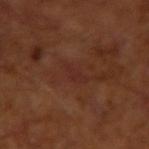No biopsy was performed on this lesion — it was imaged during a full skin examination and was not determined to be concerning. The total-body-photography lesion software estimated a lesion area of about 2.5 mm², an eccentricity of roughly 0.9, and a shape-asymmetry score of about 0.55 (0 = symmetric). And it measured border irregularity of about 6 on a 0–10 scale, a color-variation rating of about 0/10, and peripheral color asymmetry of about 0. And it measured a nevus-likeness score of about 0/100 and lesion-presence confidence of about 95/100. The lesion's longest dimension is about 2.5 mm. On the right upper arm. Captured under cross-polarized illumination. The patient is a male roughly 65 years of age. A close-up tile cropped from a whole-body skin photograph, about 15 mm across.